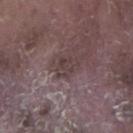workup = catalogued during a skin exam; not biopsied
illumination = white-light
acquisition = ~15 mm crop, total-body skin-cancer survey
site = the right lower leg
lesion diameter = ≈3 mm
subject = male, aged around 75